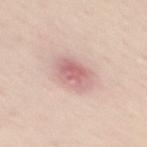Automated tile analysis of the lesion measured a lesion area of about 11 mm² and a shape eccentricity near 0.8. And it measured an automated nevus-likeness rating near 10 out of 100 and lesion-presence confidence of about 100/100.
The lesion's longest dimension is about 5 mm.
A female patient aged 28 to 32.
The tile uses white-light illumination.
The lesion is located on the mid back.
A close-up tile cropped from a whole-body skin photograph, about 15 mm across.